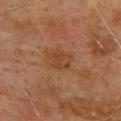follow-up: no biopsy performed (imaged during a skin exam)
subject: male, aged around 75
body site: the upper back
illumination: cross-polarized
image source: ~15 mm tile from a whole-body skin photo
automated lesion analysis: an area of roughly 5.5 mm² and a shape-asymmetry score of about 0.25 (0 = symmetric); a mean CIELAB color near L≈34 a*≈18 b*≈28, roughly 6 lightness units darker than nearby skin, and a normalized border contrast of about 5.5; a border-irregularity rating of about 2.5/10; an automated nevus-likeness rating near 0 out of 100 and a lesion-detection confidence of about 100/100
lesion size: ≈3 mm